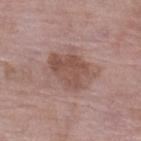Clinical impression:
The lesion was photographed on a routine skin check and not biopsied; there is no pathology result.